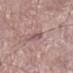biopsy_status: not biopsied; imaged during a skin examination
patient:
  sex: male
  age_approx: 65
image:
  source: total-body photography crop
  field_of_view_mm: 15
site: left lower leg